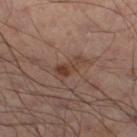Findings:
• workup: no biopsy performed (imaged during a skin exam)
• subject: male, aged 48–52
• site: the left leg
• image source: ~15 mm crop, total-body skin-cancer survey
• automated lesion analysis: an eccentricity of roughly 0.9 and two-axis asymmetry of about 0.45; a border-irregularity index near 5.5/10, internal color variation of about 3 on a 0–10 scale, and radial color variation of about 1
• illumination: cross-polarized illumination
• size: about 3.5 mm